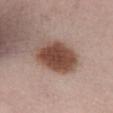notes = catalogued during a skin exam; not biopsied | automated metrics = a footprint of about 19 mm², a shape eccentricity near 0.7, and two-axis asymmetry of about 0.1 | body site = the abdomen | subject = female, about 65 years old | acquisition = 15 mm crop, total-body photography | size = ~5.5 mm (longest diameter) | illumination = white-light.